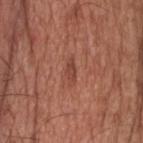A 15 mm close-up extracted from a 3D total-body photography capture. From the head or neck. The subject is a male aged 58–62.